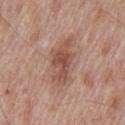Recorded during total-body skin imaging; not selected for excision or biopsy. The patient is a male roughly 70 years of age. A region of skin cropped from a whole-body photographic capture, roughly 15 mm wide. The lesion's longest dimension is about 5.5 mm. The lesion-visualizer software estimated a mean CIELAB color near L≈51 a*≈22 b*≈27, roughly 10 lightness units darker than nearby skin, and a lesion-to-skin contrast of about 7.5 (normalized; higher = more distinct). And it measured a border-irregularity index near 5.5/10 and internal color variation of about 3 on a 0–10 scale. The software also gave a lesion-detection confidence of about 100/100. The tile uses white-light illumination. From the chest.